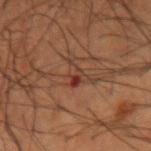follow-up = total-body-photography surveillance lesion; no biopsy | subject = male, approximately 50 years of age | size = ~2.5 mm (longest diameter) | image source = ~15 mm crop, total-body skin-cancer survey | location = the right forearm | tile lighting = cross-polarized.